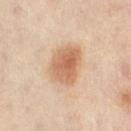Impression:
No biopsy was performed on this lesion — it was imaged during a full skin examination and was not determined to be concerning.
Image and clinical context:
Measured at roughly 5 mm in maximum diameter. Captured under cross-polarized illumination. A region of skin cropped from a whole-body photographic capture, roughly 15 mm wide. Automated tile analysis of the lesion measured an outline eccentricity of about 0.65 (0 = round, 1 = elongated) and two-axis asymmetry of about 0.15. It also reported border irregularity of about 1.5 on a 0–10 scale, internal color variation of about 5 on a 0–10 scale, and peripheral color asymmetry of about 1.5. A female patient, in their 40s. From the abdomen.